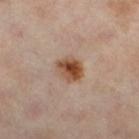Findings:
- notes — imaged on a skin check; not biopsied
- anatomic site — the left lower leg
- subject — female, aged 53 to 57
- automated lesion analysis — an average lesion color of about L≈48 a*≈20 b*≈30 (CIELAB) and a normalized lesion–skin contrast near 10.5; a classifier nevus-likeness of about 100/100 and a detector confidence of about 100 out of 100 that the crop contains a lesion
- size — about 3.5 mm
- image source — 15 mm crop, total-body photography
- tile lighting — cross-polarized illumination